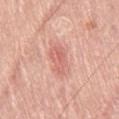Findings:
– biopsy status: no biopsy performed (imaged during a skin exam)
– anatomic site: the leg
– subject: male, aged around 80
– diameter: ≈3.5 mm
– imaging modality: total-body-photography crop, ~15 mm field of view